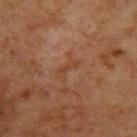Q: Is there a histopathology result?
A: no biopsy performed (imaged during a skin exam)
Q: What kind of image is this?
A: total-body-photography crop, ~15 mm field of view
Q: Automated lesion metrics?
A: an eccentricity of roughly 0.95 and two-axis asymmetry of about 0.4; a lesion–skin lightness drop of about 4; a color-variation rating of about 0/10 and radial color variation of about 0; a nevus-likeness score of about 0/100
Q: How large is the lesion?
A: about 3 mm
Q: Lesion location?
A: the upper back
Q: Who is the patient?
A: male, roughly 60 years of age
Q: What lighting was used for the tile?
A: cross-polarized illumination